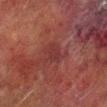Q: Is there a histopathology result?
A: total-body-photography surveillance lesion; no biopsy
Q: What did automated image analysis measure?
A: a lesion color around L≈29 a*≈24 b*≈20 in CIELAB and a normalized border contrast of about 5.5; a border-irregularity index near 2.5/10, internal color variation of about 2.5 on a 0–10 scale, and radial color variation of about 1
Q: Illumination type?
A: cross-polarized illumination
Q: Patient demographics?
A: male, aged 68–72
Q: What kind of image is this?
A: 15 mm crop, total-body photography
Q: Where on the body is the lesion?
A: the right lower leg